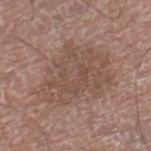{
  "biopsy_status": "not biopsied; imaged during a skin examination",
  "site": "right lower leg",
  "lighting": "white-light",
  "patient": {
    "sex": "male",
    "age_approx": 75
  },
  "image": {
    "source": "total-body photography crop",
    "field_of_view_mm": 15
  }
}